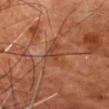Q: Was a biopsy performed?
A: catalogued during a skin exam; not biopsied
Q: Illumination type?
A: cross-polarized illumination
Q: Lesion location?
A: the chest
Q: What are the patient's age and sex?
A: male, in their 70s
Q: What did automated image analysis measure?
A: a footprint of about 6 mm², an outline eccentricity of about 0.8 (0 = round, 1 = elongated), and two-axis asymmetry of about 0.55; a mean CIELAB color near L≈43 a*≈25 b*≈33 and a lesion–skin lightness drop of about 7; a classifier nevus-likeness of about 0/100
Q: Lesion size?
A: ≈4 mm
Q: How was this image acquired?
A: 15 mm crop, total-body photography